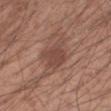Imaged during a routine full-body skin examination; the lesion was not biopsied and no histopathology is available. The patient is a male aged around 20. On the left forearm. A 15 mm close-up extracted from a 3D total-body photography capture.